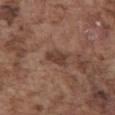Clinical impression:
The lesion was photographed on a routine skin check and not biopsied; there is no pathology result.
Context:
The lesion is located on the front of the torso. A male patient, aged 73–77. This is a white-light tile. Approximately 3 mm at its widest. A lesion tile, about 15 mm wide, cut from a 3D total-body photograph. Automated tile analysis of the lesion measured a lesion area of about 5 mm², a shape eccentricity near 0.75, and two-axis asymmetry of about 0.25. The analysis additionally found about 9 CIELAB-L* units darker than the surrounding skin and a normalized lesion–skin contrast near 7.5. The software also gave radial color variation of about 0.5. It also reported a classifier nevus-likeness of about 0/100.